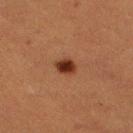Q: Was a biopsy performed?
A: catalogued during a skin exam; not biopsied
Q: What lighting was used for the tile?
A: cross-polarized illumination
Q: What kind of image is this?
A: ~15 mm crop, total-body skin-cancer survey
Q: Where on the body is the lesion?
A: the left lower leg
Q: Patient demographics?
A: female, aged around 55